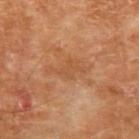  biopsy_status: not biopsied; imaged during a skin examination
  image:
    source: total-body photography crop
    field_of_view_mm: 15
  lesion_size:
    long_diameter_mm_approx: 4.5
  automated_metrics:
    area_mm2_approx: 7.0
    shape_asymmetry: 0.45
    cielab_L: 52
    cielab_a: 23
    cielab_b: 37
    vs_skin_darker_L: 6.0
    vs_skin_contrast_norm: 4.5
    border_irregularity_0_10: 6.5
    color_variation_0_10: 1.5
    peripheral_color_asymmetry: 0.5
    nevus_likeness_0_100: 0
    lesion_detection_confidence_0_100: 100
  lighting: cross-polarized
  patient:
    sex: male
    age_approx: 70
  site: arm